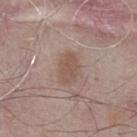Notes:
– biopsy status · total-body-photography surveillance lesion; no biopsy
– imaging modality · 15 mm crop, total-body photography
– patient · male, aged approximately 65
– illumination · white-light illumination
– body site · the chest